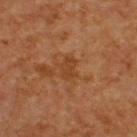Q: Was this lesion biopsied?
A: catalogued during a skin exam; not biopsied
Q: What is the anatomic site?
A: the upper back
Q: What is the imaging modality?
A: ~15 mm tile from a whole-body skin photo
Q: What did automated image analysis measure?
A: a lesion color around L≈38 a*≈22 b*≈34 in CIELAB; a border-irregularity index near 3/10 and peripheral color asymmetry of about 0.5
Q: What lighting was used for the tile?
A: cross-polarized illumination
Q: What are the patient's age and sex?
A: male, approximately 60 years of age
Q: How large is the lesion?
A: ~2.5 mm (longest diameter)